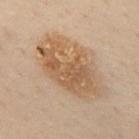Clinical impression:
Imaged during a routine full-body skin examination; the lesion was not biopsied and no histopathology is available.
Image and clinical context:
Captured under cross-polarized illumination. A male subject aged around 50. A roughly 15 mm field-of-view crop from a total-body skin photograph. The lesion's longest dimension is about 8.5 mm. Located on the mid back. Automated image analysis of the tile measured a mean CIELAB color near L≈46 a*≈14 b*≈28, a lesion–skin lightness drop of about 8, and a lesion-to-skin contrast of about 7.5 (normalized; higher = more distinct).